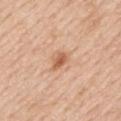- notes · no biopsy performed (imaged during a skin exam)
- image-analysis metrics · an area of roughly 3.5 mm² and two-axis asymmetry of about 0.4; an average lesion color of about L≈60 a*≈24 b*≈35 (CIELAB) and a normalized border contrast of about 7.5
- image · ~15 mm tile from a whole-body skin photo
- body site · the mid back
- lighting · white-light illumination
- patient · male, approximately 60 years of age